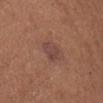Q: Is there a histopathology result?
A: total-body-photography surveillance lesion; no biopsy
Q: How was the tile lit?
A: white-light illumination
Q: How was this image acquired?
A: ~15 mm tile from a whole-body skin photo
Q: Patient demographics?
A: male, approximately 65 years of age
Q: Lesion location?
A: the chest